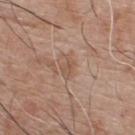Clinical impression: No biopsy was performed on this lesion — it was imaged during a full skin examination and was not determined to be concerning. Clinical summary: The lesion is on the chest. An algorithmic analysis of the crop reported a lesion color around L≈53 a*≈18 b*≈28 in CIELAB. The software also gave a border-irregularity index near 4.5/10, a within-lesion color-variation index near 1.5/10, and peripheral color asymmetry of about 0.5. It also reported a detector confidence of about 100 out of 100 that the crop contains a lesion. Cropped from a whole-body photographic skin survey; the tile spans about 15 mm. Captured under white-light illumination. A male subject approximately 75 years of age.